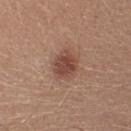Assessment:
This lesion was catalogued during total-body skin photography and was not selected for biopsy.
Image and clinical context:
From the head or neck. This is a white-light tile. About 3.5 mm across. The subject is a female aged 28 to 32. A 15 mm crop from a total-body photograph taken for skin-cancer surveillance.